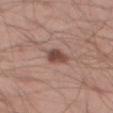No biopsy was performed on this lesion — it was imaged during a full skin examination and was not determined to be concerning. Automated tile analysis of the lesion measured an eccentricity of roughly 0.75 and two-axis asymmetry of about 0.25. The analysis additionally found roughly 13 lightness units darker than nearby skin and a lesion-to-skin contrast of about 9.5 (normalized; higher = more distinct). It also reported border irregularity of about 2 on a 0–10 scale, internal color variation of about 3.5 on a 0–10 scale, and a peripheral color-asymmetry measure near 1. The tile uses white-light illumination. Longest diameter approximately 3 mm. A 15 mm crop from a total-body photograph taken for skin-cancer surveillance. A male subject about 40 years old. From the left thigh.